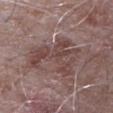This lesion was catalogued during total-body skin photography and was not selected for biopsy.
A lesion tile, about 15 mm wide, cut from a 3D total-body photograph.
A male patient approximately 65 years of age.
Located on the left upper arm.
The lesion-visualizer software estimated an area of roughly 19 mm² and a shape-asymmetry score of about 0.6 (0 = symmetric). The software also gave an average lesion color of about L≈44 a*≈18 b*≈19 (CIELAB), a lesion–skin lightness drop of about 8, and a normalized border contrast of about 6.5. The software also gave a border-irregularity index near 9.5/10 and a color-variation rating of about 4/10. It also reported a nevus-likeness score of about 0/100 and a lesion-detection confidence of about 95/100.
This is a white-light tile.
Approximately 7 mm at its widest.